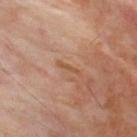{"biopsy_status": "not biopsied; imaged during a skin examination", "lighting": "cross-polarized", "image": {"source": "total-body photography crop", "field_of_view_mm": 15}, "lesion_size": {"long_diameter_mm_approx": 2.5}, "site": "upper back", "patient": {"sex": "male", "age_approx": 50}}